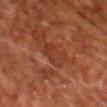This lesion was catalogued during total-body skin photography and was not selected for biopsy. A male patient, aged approximately 60. A close-up tile cropped from a whole-body skin photograph, about 15 mm across. This is a cross-polarized tile. The lesion's longest dimension is about 3.5 mm. The lesion is on the chest.